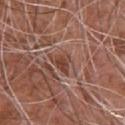Q: Was a biopsy performed?
A: total-body-photography surveillance lesion; no biopsy
Q: Patient demographics?
A: male, aged 73 to 77
Q: Illumination type?
A: white-light illumination
Q: Lesion location?
A: the chest
Q: How was this image acquired?
A: total-body-photography crop, ~15 mm field of view
Q: Lesion size?
A: about 2.5 mm
Q: Automated lesion metrics?
A: an area of roughly 4.5 mm², an outline eccentricity of about 0.5 (0 = round, 1 = elongated), and a shape-asymmetry score of about 0.45 (0 = symmetric); an average lesion color of about L≈43 a*≈22 b*≈27 (CIELAB) and roughly 10 lightness units darker than nearby skin; a classifier nevus-likeness of about 0/100